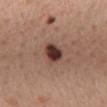Recorded during total-body skin imaging; not selected for excision or biopsy.
A female subject about 55 years old.
The lesion is on the mid back.
Approximately 2.5 mm at its widest.
A lesion tile, about 15 mm wide, cut from a 3D total-body photograph.
Imaged with white-light lighting.
The lesion-visualizer software estimated a lesion area of about 5.5 mm². And it measured a nevus-likeness score of about 90/100.